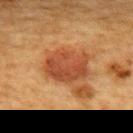Assessment: This lesion was catalogued during total-body skin photography and was not selected for biopsy. Background: Measured at roughly 5 mm in maximum diameter. The lesion-visualizer software estimated roughly 10 lightness units darker than nearby skin and a lesion-to-skin contrast of about 8 (normalized; higher = more distinct). The analysis additionally found border irregularity of about 2 on a 0–10 scale and radial color variation of about 1.5. And it measured a nevus-likeness score of about 100/100. This image is a 15 mm lesion crop taken from a total-body photograph. The patient is a female aged 38 to 42. From the mid back.